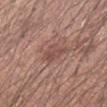notes = imaged on a skin check; not biopsied | subject = male, roughly 30 years of age | illumination = white-light illumination | anatomic site = the left forearm | acquisition = 15 mm crop, total-body photography | size = ~2.5 mm (longest diameter).